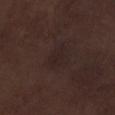Acquisition and patient details: Captured under white-light illumination. The lesion is located on the leg. A 15 mm close-up tile from a total-body photography series done for melanoma screening. The patient is a male about 70 years old. Automated tile analysis of the lesion measured a footprint of about 5 mm² and a shape eccentricity near 0.85. It also reported a lesion color around L≈21 a*≈12 b*≈14 in CIELAB and roughly 3 lightness units darker than nearby skin. The recorded lesion diameter is about 3.5 mm.